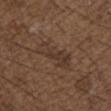Case summary:
- biopsy status · catalogued during a skin exam; not biopsied
- diameter · ~5.5 mm (longest diameter)
- lighting · white-light
- patient · male, about 50 years old
- imaging modality · total-body-photography crop, ~15 mm field of view
- body site · the chest